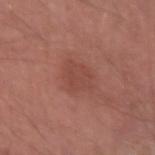Context:
Cropped from a total-body skin-imaging series; the visible field is about 15 mm. Longest diameter approximately 4 mm. Located on the right forearm. A male subject, aged approximately 55.